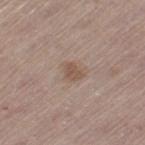Case summary:
– biopsy status — no biopsy performed (imaged during a skin exam)
– image source — 15 mm crop, total-body photography
– patient — male, roughly 65 years of age
– body site — the leg